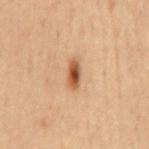This lesion was catalogued during total-body skin photography and was not selected for biopsy. The tile uses cross-polarized illumination. A close-up tile cropped from a whole-body skin photograph, about 15 mm across. An algorithmic analysis of the crop reported an average lesion color of about L≈53 a*≈23 b*≈36 (CIELAB), a lesion–skin lightness drop of about 15, and a normalized lesion–skin contrast near 10.5. It also reported an automated nevus-likeness rating near 100 out of 100 and a lesion-detection confidence of about 100/100. The lesion's longest dimension is about 3.5 mm. A male patient in their 60s. On the mid back.